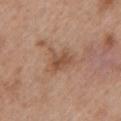Imaged with white-light lighting. From the arm. The subject is a female about 40 years old. The recorded lesion diameter is about 3.5 mm. Cropped from a total-body skin-imaging series; the visible field is about 15 mm. The total-body-photography lesion software estimated a footprint of about 6.5 mm² and two-axis asymmetry of about 0.45. And it measured a border-irregularity index near 5/10 and a within-lesion color-variation index near 3/10. It also reported a classifier nevus-likeness of about 0/100 and a detector confidence of about 100 out of 100 that the crop contains a lesion.